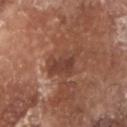biopsy status=catalogued during a skin exam; not biopsied | acquisition=~15 mm crop, total-body skin-cancer survey | anatomic site=the head or neck | size=about 4.5 mm | tile lighting=white-light | patient=male, aged 78 to 82.